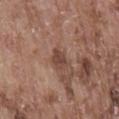No biopsy was performed on this lesion — it was imaged during a full skin examination and was not determined to be concerning.
Measured at roughly 2.5 mm in maximum diameter.
An algorithmic analysis of the crop reported a shape eccentricity near 0.6 and a shape-asymmetry score of about 0.25 (0 = symmetric). The analysis additionally found internal color variation of about 2 on a 0–10 scale.
A 15 mm close-up extracted from a 3D total-body photography capture.
Captured under white-light illumination.
A male patient aged 73–77.
The lesion is located on the lower back.